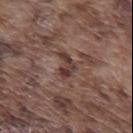Q: Was this lesion biopsied?
A: no biopsy performed (imaged during a skin exam)
Q: Patient demographics?
A: male, aged approximately 75
Q: How large is the lesion?
A: ~3.5 mm (longest diameter)
Q: Lesion location?
A: the mid back
Q: Illumination type?
A: white-light illumination
Q: What kind of image is this?
A: ~15 mm crop, total-body skin-cancer survey